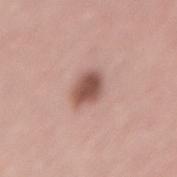Clinical impression:
Part of a total-body skin-imaging series; this lesion was reviewed on a skin check and was not flagged for biopsy.
Clinical summary:
Located on the lower back. The recorded lesion diameter is about 3.5 mm. Cropped from a whole-body photographic skin survey; the tile spans about 15 mm. A male patient aged approximately 45.